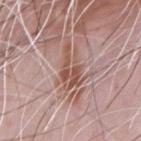Part of a total-body skin-imaging series; this lesion was reviewed on a skin check and was not flagged for biopsy. This image is a 15 mm lesion crop taken from a total-body photograph. On the chest. This is a white-light tile. A male patient approximately 70 years of age. Longest diameter approximately 5 mm.